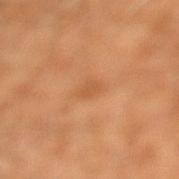<case>
  <lighting>cross-polarized</lighting>
  <patient>
    <sex>male</sex>
    <age_approx>30</age_approx>
  </patient>
  <image>
    <source>total-body photography crop</source>
    <field_of_view_mm>15</field_of_view_mm>
  </image>
  <site>left lower leg</site>
  <lesion_size>
    <long_diameter_mm_approx>2.5</long_diameter_mm_approx>
  </lesion_size>
</case>